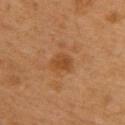Notes:
• biopsy status: imaged on a skin check; not biopsied
• patient: male, approximately 55 years of age
• body site: the upper back
• automated lesion analysis: a footprint of about 4.5 mm², a shape eccentricity near 0.5, and two-axis asymmetry of about 0.25; border irregularity of about 2.5 on a 0–10 scale and a within-lesion color-variation index near 1.5/10; an automated nevus-likeness rating near 25 out of 100 and a lesion-detection confidence of about 100/100
• illumination: cross-polarized illumination
• image: 15 mm crop, total-body photography
• lesion size: ≈2.5 mm